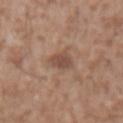patient: male, aged approximately 45 | diameter: ~3 mm (longest diameter) | lighting: white-light illumination | body site: the abdomen | acquisition: 15 mm crop, total-body photography.